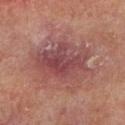{
  "biopsy_status": "not biopsied; imaged during a skin examination",
  "site": "left lower leg",
  "image": {
    "source": "total-body photography crop",
    "field_of_view_mm": 15
  },
  "patient": {
    "sex": "male",
    "age_approx": 65
  }
}